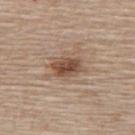Q: Was a biopsy performed?
A: imaged on a skin check; not biopsied
Q: What kind of image is this?
A: total-body-photography crop, ~15 mm field of view
Q: How was the tile lit?
A: white-light illumination
Q: What are the patient's age and sex?
A: female, aged approximately 65
Q: What is the anatomic site?
A: the back